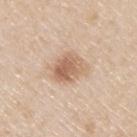Findings:
* biopsy status · imaged on a skin check; not biopsied
* image · ~15 mm crop, total-body skin-cancer survey
* lighting · white-light illumination
* location · the upper back
* subject · male, about 45 years old
* automated lesion analysis · an automated nevus-likeness rating near 65 out of 100